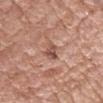Findings:
– workup · catalogued during a skin exam; not biopsied